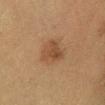biopsy_status: not biopsied; imaged during a skin examination
patient:
  sex: female
  age_approx: 40
site: left lower leg
lighting: cross-polarized
lesion_size:
  long_diameter_mm_approx: 4.0
image:
  source: total-body photography crop
  field_of_view_mm: 15